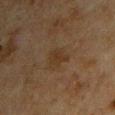Clinical summary:
This is a cross-polarized tile. Located on the upper back. A male patient aged approximately 65. Measured at roughly 3 mm in maximum diameter. The total-body-photography lesion software estimated an eccentricity of roughly 0.7 and a symmetry-axis asymmetry near 0.25. The software also gave a mean CIELAB color near L≈26 a*≈12 b*≈24 and roughly 5 lightness units darker than nearby skin. A 15 mm close-up tile from a total-body photography series done for melanoma screening.